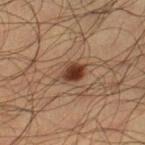follow-up — no biopsy performed (imaged during a skin exam).